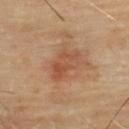follow-up: catalogued during a skin exam; not biopsied | anatomic site: the back | imaging modality: ~15 mm crop, total-body skin-cancer survey | subject: male, in their mid-60s.